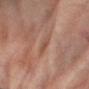Q: Was this lesion biopsied?
A: catalogued during a skin exam; not biopsied
Q: What kind of image is this?
A: 15 mm crop, total-body photography
Q: Where on the body is the lesion?
A: the right forearm
Q: Patient demographics?
A: female, aged around 80
Q: How large is the lesion?
A: about 3 mm
Q: How was the tile lit?
A: cross-polarized illumination
Q: What did automated image analysis measure?
A: an eccentricity of roughly 0.9 and a shape-asymmetry score of about 0.2 (0 = symmetric); a lesion color around L≈43 a*≈19 b*≈25 in CIELAB and a normalized lesion–skin contrast near 5; a color-variation rating of about 1.5/10 and a peripheral color-asymmetry measure near 0.5; a nevus-likeness score of about 0/100 and lesion-presence confidence of about 95/100